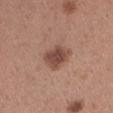* site — the leg
* automated lesion analysis — a lesion area of about 8.5 mm² and a shape-asymmetry score of about 0.25 (0 = symmetric); a mean CIELAB color near L≈47 a*≈21 b*≈26 and a lesion–skin lightness drop of about 12; a border-irregularity rating of about 2/10, a within-lesion color-variation index near 2.5/10, and a peripheral color-asymmetry measure near 1; lesion-presence confidence of about 100/100
* illumination — white-light illumination
* subject — female, about 30 years old
* image — 15 mm crop, total-body photography